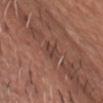Image and clinical context:
On the chest. The subject is a male roughly 75 years of age. This is a white-light tile. The lesion's longest dimension is about 2.5 mm. A 15 mm close-up tile from a total-body photography series done for melanoma screening.